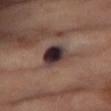Q: Is there a histopathology result?
A: imaged on a skin check; not biopsied
Q: What kind of image is this?
A: 15 mm crop, total-body photography
Q: Lesion location?
A: the abdomen
Q: What are the patient's age and sex?
A: female, aged around 55